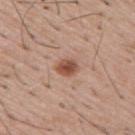No biopsy was performed on this lesion — it was imaged during a full skin examination and was not determined to be concerning. The patient is a male roughly 55 years of age. The lesion-visualizer software estimated roughly 13 lightness units darker than nearby skin and a lesion-to-skin contrast of about 9 (normalized; higher = more distinct). Imaged with white-light lighting. This image is a 15 mm lesion crop taken from a total-body photograph. Longest diameter approximately 2.5 mm.